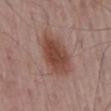From the mid back. The lesion's longest dimension is about 5.5 mm. A region of skin cropped from a whole-body photographic capture, roughly 15 mm wide. The subject is a male aged 63–67.